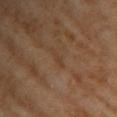The lesion was tiled from a total-body skin photograph and was not biopsied. The lesion is located on the arm. Captured under cross-polarized illumination. About 2.5 mm across. A 15 mm crop from a total-body photograph taken for skin-cancer surveillance. A female patient roughly 65 years of age.